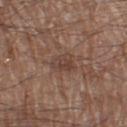No biopsy was performed on this lesion — it was imaged during a full skin examination and was not determined to be concerning. Captured under white-light illumination. A 15 mm close-up extracted from a 3D total-body photography capture. The subject is a male aged 78–82. An algorithmic analysis of the crop reported an eccentricity of roughly 0.5 and a symmetry-axis asymmetry near 0.4. And it measured an average lesion color of about L≈41 a*≈17 b*≈24 (CIELAB), roughly 7 lightness units darker than nearby skin, and a normalized lesion–skin contrast near 6. It also reported an automated nevus-likeness rating near 0 out of 100 and a detector confidence of about 100 out of 100 that the crop contains a lesion. On the leg.